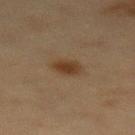automated lesion analysis=a mean CIELAB color near L≈32 a*≈14 b*≈27, a lesion–skin lightness drop of about 8, and a normalized lesion–skin contrast near 8.5; border irregularity of about 2 on a 0–10 scale and a peripheral color-asymmetry measure near 0.5; an automated nevus-likeness rating near 100 out of 100 and lesion-presence confidence of about 100/100
subject=female, aged 38 to 42
location=the leg
image source=15 mm crop, total-body photography
lesion diameter=≈3 mm